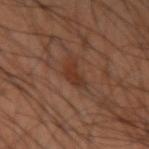Impression:
This lesion was catalogued during total-body skin photography and was not selected for biopsy.
Clinical summary:
A region of skin cropped from a whole-body photographic capture, roughly 15 mm wide. A male subject aged 38–42. The lesion is on the left upper arm.